The lesion's longest dimension is about 7.5 mm. The lesion is on the upper back. A male subject in their mid-50s. A 15 mm close-up tile from a total-body photography series done for melanoma screening.
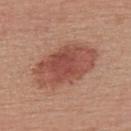Diagnosis:
Biopsy histopathology demonstrated a dysplastic (Clark) nevus.